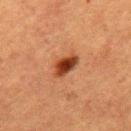Assessment:
Imaged during a routine full-body skin examination; the lesion was not biopsied and no histopathology is available.
Background:
Approximately 3.5 mm at its widest. The lesion is located on the mid back. A male subject aged around 50. This is a cross-polarized tile. A 15 mm crop from a total-body photograph taken for skin-cancer surveillance.